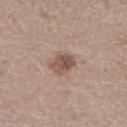image source: ~15 mm crop, total-body skin-cancer survey
lighting: white-light
image-analysis metrics: a lesion area of about 5.5 mm², an outline eccentricity of about 0.7 (0 = round, 1 = elongated), and a symmetry-axis asymmetry near 0.2; an average lesion color of about L≈52 a*≈18 b*≈25 (CIELAB), a lesion–skin lightness drop of about 12, and a normalized lesion–skin contrast near 8.5; a color-variation rating of about 4.5/10
site: the right lower leg
subject: male, about 50 years old